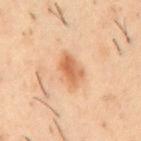Part of a total-body skin-imaging series; this lesion was reviewed on a skin check and was not flagged for biopsy. A roughly 15 mm field-of-view crop from a total-body skin photograph. About 4 mm across. Captured under cross-polarized illumination. The lesion is located on the mid back. A male patient aged 53 to 57.